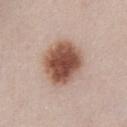Clinical impression:
No biopsy was performed on this lesion — it was imaged during a full skin examination and was not determined to be concerning.
Clinical summary:
A roughly 15 mm field-of-view crop from a total-body skin photograph. The patient is a female in their 40s. From the chest. Measured at roughly 5.5 mm in maximum diameter.